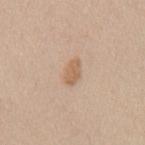notes: catalogued during a skin exam; not biopsied
image: total-body-photography crop, ~15 mm field of view
subject: female, approximately 40 years of age
illumination: white-light illumination
TBP lesion metrics: an area of roughly 4 mm², an eccentricity of roughly 0.85, and a shape-asymmetry score of about 0.25 (0 = symmetric)
site: the mid back
lesion size: about 3 mm